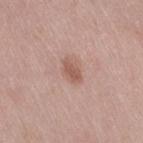{
  "biopsy_status": "not biopsied; imaged during a skin examination",
  "lesion_size": {
    "long_diameter_mm_approx": 3.0
  },
  "site": "leg",
  "automated_metrics": {
    "area_mm2_approx": 3.5,
    "eccentricity": 0.8,
    "shape_asymmetry": 0.25,
    "cielab_L": 56,
    "cielab_a": 21,
    "cielab_b": 27,
    "vs_skin_darker_L": 9.0,
    "vs_skin_contrast_norm": 7.0,
    "border_irregularity_0_10": 2.5,
    "color_variation_0_10": 1.0,
    "peripheral_color_asymmetry": 0.5,
    "nevus_likeness_0_100": 40,
    "lesion_detection_confidence_0_100": 100
  },
  "patient": {
    "sex": "female",
    "age_approx": 40
  },
  "image": {
    "source": "total-body photography crop",
    "field_of_view_mm": 15
  },
  "lighting": "white-light"
}